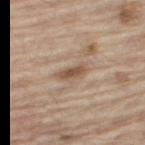<record>
<site>leg</site>
<lighting>white-light</lighting>
<image>
  <source>total-body photography crop</source>
  <field_of_view_mm>15</field_of_view_mm>
</image>
<patient>
  <sex>male</sex>
  <age_approx>70</age_approx>
</patient>
</record>